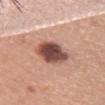The lesion was tiled from a total-body skin photograph and was not biopsied. The tile uses white-light illumination. A female patient roughly 60 years of age. From the front of the torso. A roughly 15 mm field-of-view crop from a total-body skin photograph. Measured at roughly 5 mm in maximum diameter.